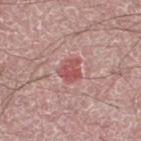No biopsy was performed on this lesion — it was imaged during a full skin examination and was not determined to be concerning. The lesion's longest dimension is about 3 mm. Imaged with white-light lighting. The total-body-photography lesion software estimated an area of roughly 5 mm² and a shape eccentricity near 0.5. It also reported an automated nevus-likeness rating near 15 out of 100 and a detector confidence of about 100 out of 100 that the crop contains a lesion. The lesion is on the leg. A male subject, aged 48 to 52. A roughly 15 mm field-of-view crop from a total-body skin photograph.